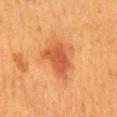patient:
  sex: female
  age_approx: 50
image:
  source: total-body photography crop
  field_of_view_mm: 15
lesion_size:
  long_diameter_mm_approx: 4.5
automated_metrics:
  area_mm2_approx: 10.0
  color_variation_0_10: 2.5
  nevus_likeness_0_100: 95
  lesion_detection_confidence_0_100: 100
site: back